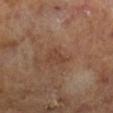Notes:
– image-analysis metrics: a lesion area of about 3 mm², an outline eccentricity of about 0.85 (0 = round, 1 = elongated), and two-axis asymmetry of about 0.3; a lesion color around L≈40 a*≈19 b*≈27 in CIELAB, about 5 CIELAB-L* units darker than the surrounding skin, and a lesion-to-skin contrast of about 4.5 (normalized; higher = more distinct)
– lesion size: about 2.5 mm
– image source: 15 mm crop, total-body photography
– subject: male, in their mid-60s
– lighting: cross-polarized illumination